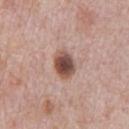The subject is a male about 70 years old. Located on the chest. The tile uses white-light illumination. Approximately 3.5 mm at its widest. A roughly 15 mm field-of-view crop from a total-body skin photograph.